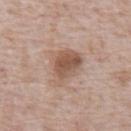  biopsy_status: not biopsied; imaged during a skin examination
  image:
    source: total-body photography crop
    field_of_view_mm: 15
  site: abdomen
  lighting: white-light
  lesion_size:
    long_diameter_mm_approx: 5.0
  patient:
    sex: male
    age_approx: 70